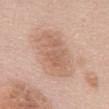Background:
A 15 mm crop from a total-body photograph taken for skin-cancer surveillance. The patient is a female about 60 years old. Located on the mid back. Automated image analysis of the tile measured a lesion color around L≈63 a*≈19 b*≈29 in CIELAB, about 8 CIELAB-L* units darker than the surrounding skin, and a normalized lesion–skin contrast near 5.5. It also reported lesion-presence confidence of about 100/100. Longest diameter approximately 6.5 mm. This is a white-light tile.